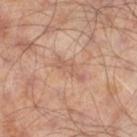Imaged during a routine full-body skin examination; the lesion was not biopsied and no histopathology is available. A male patient aged 63–67. Cropped from a whole-body photographic skin survey; the tile spans about 15 mm. Located on the right thigh. The lesion's longest dimension is about 4 mm.